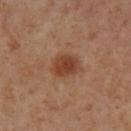This lesion was catalogued during total-body skin photography and was not selected for biopsy.
A region of skin cropped from a whole-body photographic capture, roughly 15 mm wide.
This is a cross-polarized tile.
From the left lower leg.
Measured at roughly 3.5 mm in maximum diameter.
The total-body-photography lesion software estimated a nevus-likeness score of about 95/100 and lesion-presence confidence of about 100/100.
The patient is a female aged around 55.